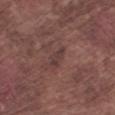Q: What are the patient's age and sex?
A: male, in their mid-70s
Q: How large is the lesion?
A: about 3 mm
Q: How was this image acquired?
A: 15 mm crop, total-body photography
Q: How was the tile lit?
A: white-light
Q: Where on the body is the lesion?
A: the left forearm